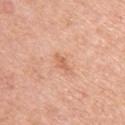Assessment:
The lesion was tiled from a total-body skin photograph and was not biopsied.
Image and clinical context:
A region of skin cropped from a whole-body photographic capture, roughly 15 mm wide. Measured at roughly 2.5 mm in maximum diameter. The lesion is located on the left upper arm. A female patient about 60 years old.